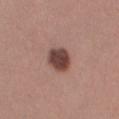<case>
  <automated_metrics>
    <cielab_L>43</cielab_L>
    <cielab_a>20</cielab_a>
    <cielab_b>22</cielab_b>
    <vs_skin_darker_L>16.0</vs_skin_darker_L>
    <vs_skin_contrast_norm>11.5</vs_skin_contrast_norm>
  </automated_metrics>
  <image>
    <source>total-body photography crop</source>
    <field_of_view_mm>15</field_of_view_mm>
  </image>
  <patient>
    <sex>female</sex>
    <age_approx>25</age_approx>
  </patient>
  <lighting>white-light</lighting>
  <lesion_size>
    <long_diameter_mm_approx>3.5</long_diameter_mm_approx>
  </lesion_size>
  <site>left thigh</site>
</case>